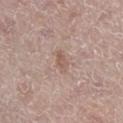notes = total-body-photography surveillance lesion; no biopsy.